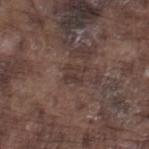Notes:
* follow-up · catalogued during a skin exam; not biopsied
* lighting · white-light
* image source · 15 mm crop, total-body photography
* lesion size · about 2.5 mm
* subject · male, in their mid-70s
* image-analysis metrics · a nevus-likeness score of about 0/100
* anatomic site · the right lower leg